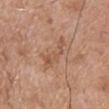workup=no biopsy performed (imaged during a skin exam) | illumination=white-light | body site=the chest | image=total-body-photography crop, ~15 mm field of view | subject=male, aged 63 to 67.